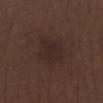Part of a total-body skin-imaging series; this lesion was reviewed on a skin check and was not flagged for biopsy.
The lesion's longest dimension is about 4.5 mm.
A male subject roughly 70 years of age.
Imaged with white-light lighting.
The lesion is located on the left lower leg.
A lesion tile, about 15 mm wide, cut from a 3D total-body photograph.